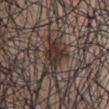A 15 mm crop from a total-body photograph taken for skin-cancer surveillance.
The lesion is located on the chest.
The subject is a male approximately 70 years of age.